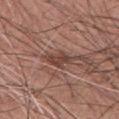The lesion was tiled from a total-body skin photograph and was not biopsied. Approximately 4.5 mm at its widest. The lesion is located on the chest. Automated tile analysis of the lesion measured a shape-asymmetry score of about 0.35 (0 = symmetric). It also reported a border-irregularity rating of about 4.5/10 and a color-variation rating of about 2/10. A male subject, roughly 55 years of age. Cropped from a total-body skin-imaging series; the visible field is about 15 mm.